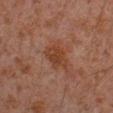Clinical impression: Part of a total-body skin-imaging series; this lesion was reviewed on a skin check and was not flagged for biopsy. Clinical summary: The patient is a male roughly 30 years of age. Located on the arm. The lesion's longest dimension is about 3.5 mm. The tile uses cross-polarized illumination. A 15 mm crop from a total-body photograph taken for skin-cancer surveillance.